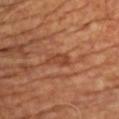biopsy_status: not biopsied; imaged during a skin examination
image:
  source: total-body photography crop
  field_of_view_mm: 15
site: chest
patient:
  sex: male
  age_approx: 55